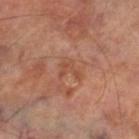Q: Is there a histopathology result?
A: imaged on a skin check; not biopsied
Q: Who is the patient?
A: male, aged around 70
Q: What is the lesion's diameter?
A: ~2.5 mm (longest diameter)
Q: Illumination type?
A: cross-polarized
Q: Where on the body is the lesion?
A: the right lower leg
Q: What is the imaging modality?
A: total-body-photography crop, ~15 mm field of view